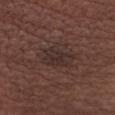biopsy status — imaged on a skin check; not biopsied | location — the abdomen | image source — 15 mm crop, total-body photography | patient — male, aged 38 to 42.